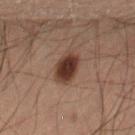Q: How large is the lesion?
A: about 3.5 mm
Q: What kind of image is this?
A: ~15 mm crop, total-body skin-cancer survey
Q: What is the anatomic site?
A: the right thigh
Q: Patient demographics?
A: male, aged 58–62
Q: Illumination type?
A: cross-polarized illumination